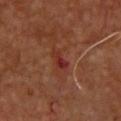The lesion is on the chest. This is a cross-polarized tile. About 3 mm across. The patient is a male aged approximately 50. A region of skin cropped from a whole-body photographic capture, roughly 15 mm wide.